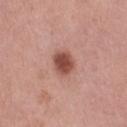{
  "patient": {
    "sex": "female",
    "age_approx": 40
  },
  "lesion_size": {
    "long_diameter_mm_approx": 3.0
  },
  "lighting": "white-light",
  "image": {
    "source": "total-body photography crop",
    "field_of_view_mm": 15
  },
  "site": "right thigh"
}